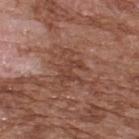* follow-up · imaged on a skin check; not biopsied
* illumination · white-light illumination
* lesion size · ≈4.5 mm
* image source · 15 mm crop, total-body photography
* patient · male, aged 73 to 77
* anatomic site · the upper back